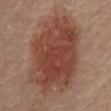follow-up=catalogued during a skin exam; not biopsied | subject=male, in their mid- to late 80s or older | automated lesion analysis=a mean CIELAB color near L≈41 a*≈21 b*≈26 and a normalized lesion–skin contrast near 9; a border-irregularity rating of about 3/10, a within-lesion color-variation index near 4.5/10, and radial color variation of about 1.5; a classifier nevus-likeness of about 100/100 and a detector confidence of about 100 out of 100 that the crop contains a lesion | image=~15 mm crop, total-body skin-cancer survey | location=the mid back.